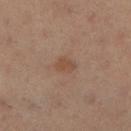Findings:
– workup — imaged on a skin check; not biopsied
– lesion size — ~2.5 mm (longest diameter)
– tile lighting — cross-polarized illumination
– body site — the right lower leg
– image source — ~15 mm tile from a whole-body skin photo
– patient — female, in their mid- to late 30s